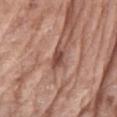  biopsy_status: not biopsied; imaged during a skin examination
  lighting: white-light
  site: right forearm
  lesion_size:
    long_diameter_mm_approx: 3.0
  patient:
    sex: female
    age_approx: 75
  image:
    source: total-body photography crop
    field_of_view_mm: 15